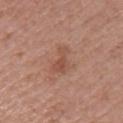Assessment: The lesion was photographed on a routine skin check and not biopsied; there is no pathology result. Clinical summary: The lesion is on the right upper arm. A female subject aged 48–52. A roughly 15 mm field-of-view crop from a total-body skin photograph. An algorithmic analysis of the crop reported a nevus-likeness score of about 5/100 and a detector confidence of about 100 out of 100 that the crop contains a lesion. Longest diameter approximately 3.5 mm.